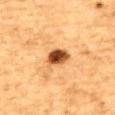Findings:
* follow-up · catalogued during a skin exam; not biopsied
* diameter · about 3 mm
* anatomic site · the upper back
* patient · male, in their mid- to late 80s or older
* acquisition · ~15 mm tile from a whole-body skin photo